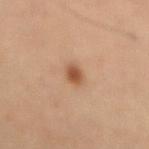Assessment: The lesion was photographed on a routine skin check and not biopsied; there is no pathology result.